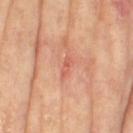Assessment: No biopsy was performed on this lesion — it was imaged during a full skin examination and was not determined to be concerning. Image and clinical context: Imaged with cross-polarized lighting. Longest diameter approximately 2.5 mm. A 15 mm close-up extracted from a 3D total-body photography capture. The lesion is on the right thigh. A female patient aged approximately 75. Automated image analysis of the tile measured a footprint of about 2 mm² and an outline eccentricity of about 0.95 (0 = round, 1 = elongated). It also reported a border-irregularity index near 3.5/10 and a color-variation rating of about 0/10. And it measured a lesion-detection confidence of about 100/100.